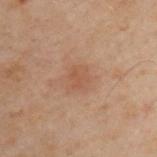biopsy status = imaged on a skin check; not biopsied
image = ~15 mm crop, total-body skin-cancer survey
subject = male, roughly 50 years of age
body site = the upper back
size = ~2.5 mm (longest diameter)
illumination = cross-polarized illumination